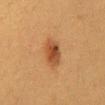Q: Is there a histopathology result?
A: total-body-photography surveillance lesion; no biopsy
Q: What did automated image analysis measure?
A: an average lesion color of about L≈40 a*≈21 b*≈32 (CIELAB) and a lesion–skin lightness drop of about 10; internal color variation of about 4 on a 0–10 scale and radial color variation of about 1; an automated nevus-likeness rating near 100 out of 100 and a detector confidence of about 100 out of 100 that the crop contains a lesion
Q: What is the imaging modality?
A: total-body-photography crop, ~15 mm field of view
Q: Lesion size?
A: about 4 mm
Q: Patient demographics?
A: female, aged 38–42
Q: Where on the body is the lesion?
A: the chest